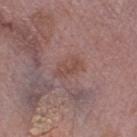Imaged during a routine full-body skin examination; the lesion was not biopsied and no histopathology is available. Cropped from a whole-body photographic skin survey; the tile spans about 15 mm. The lesion is on the left thigh. The tile uses white-light illumination. Approximately 3.5 mm at its widest. The lesion-visualizer software estimated a lesion area of about 5 mm², an eccentricity of roughly 0.85, and two-axis asymmetry of about 0.25. The analysis additionally found border irregularity of about 3.5 on a 0–10 scale, a color-variation rating of about 1.5/10, and a peripheral color-asymmetry measure near 0.5. The software also gave a lesion-detection confidence of about 100/100. A female subject aged approximately 70.